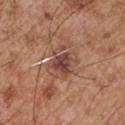Recorded during total-body skin imaging; not selected for excision or biopsy. A region of skin cropped from a whole-body photographic capture, roughly 15 mm wide. About 4 mm across. Automated tile analysis of the lesion measured a footprint of about 7.5 mm² and an eccentricity of roughly 0.7. The analysis additionally found internal color variation of about 3.5 on a 0–10 scale and peripheral color asymmetry of about 1. It also reported an automated nevus-likeness rating near 0 out of 100. This is a white-light tile. From the right upper arm. A male patient aged around 55.